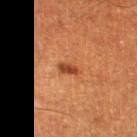Case summary:
– notes — no biopsy performed (imaged during a skin exam)
– body site — the left thigh
– lesion size — ~2.5 mm (longest diameter)
– lighting — cross-polarized illumination
– acquisition — total-body-photography crop, ~15 mm field of view
– subject — male, approximately 60 years of age